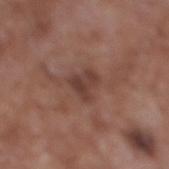Clinical impression: The lesion was tiled from a total-body skin photograph and was not biopsied. Context: The patient is a male aged 58 to 62. This is a white-light tile. The lesion is on the left lower leg. A close-up tile cropped from a whole-body skin photograph, about 15 mm across. The total-body-photography lesion software estimated a lesion–skin lightness drop of about 9.